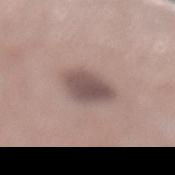follow-up: total-body-photography surveillance lesion; no biopsy
diameter: ~3.5 mm (longest diameter)
location: the left lower leg
image-analysis metrics: an area of roughly 10 mm², a shape eccentricity near 0.45, and a symmetry-axis asymmetry near 0.2; about 12 CIELAB-L* units darker than the surrounding skin; radial color variation of about 1
image source: total-body-photography crop, ~15 mm field of view
patient: female, aged around 60